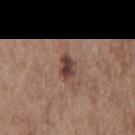Captured during whole-body skin photography for melanoma surveillance; the lesion was not biopsied.